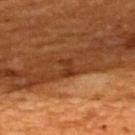notes — total-body-photography surveillance lesion; no biopsy | anatomic site — the upper back | subject — male, approximately 60 years of age | acquisition — ~15 mm crop, total-body skin-cancer survey.